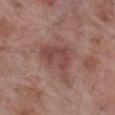workup: catalogued during a skin exam; not biopsied
subject: male, aged approximately 70
size: ~5.5 mm (longest diameter)
automated metrics: an area of roughly 11 mm² and a shape-asymmetry score of about 0.45 (0 = symmetric); a lesion–skin lightness drop of about 8 and a normalized border contrast of about 6.5; an automated nevus-likeness rating near 0 out of 100 and a lesion-detection confidence of about 100/100
image source: ~15 mm crop, total-body skin-cancer survey
site: the right lower leg